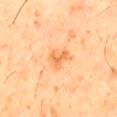Recorded during total-body skin imaging; not selected for excision or biopsy. Located on the right thigh. Automated image analysis of the tile measured border irregularity of about 4.5 on a 0–10 scale, a color-variation rating of about 2.5/10, and radial color variation of about 1. A 15 mm close-up extracted from a 3D total-body photography capture. The lesion's longest dimension is about 3 mm. This is a cross-polarized tile. A male patient aged approximately 60.